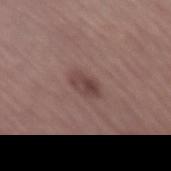Impression:
Recorded during total-body skin imaging; not selected for excision or biopsy.
Acquisition and patient details:
A female subject roughly 50 years of age. Cropped from a whole-body photographic skin survey; the tile spans about 15 mm. The tile uses white-light illumination. The lesion is on the left thigh. Automated tile analysis of the lesion measured a lesion area of about 5 mm² and an outline eccentricity of about 0.8 (0 = round, 1 = elongated). The analysis additionally found roughly 9 lightness units darker than nearby skin and a normalized lesion–skin contrast near 7. The analysis additionally found a border-irregularity rating of about 1.5/10, a color-variation rating of about 4/10, and peripheral color asymmetry of about 1.5. The recorded lesion diameter is about 3.5 mm.